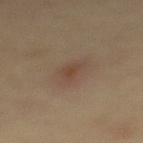Captured during whole-body skin photography for melanoma surveillance; the lesion was not biopsied. Cropped from a whole-body photographic skin survey; the tile spans about 15 mm. Measured at roughly 3 mm in maximum diameter. On the upper back. The patient is a female roughly 65 years of age.